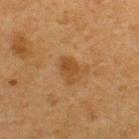A male patient in their mid-70s. The lesion is located on the upper back. Cropped from a whole-body photographic skin survey; the tile spans about 15 mm.